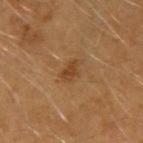No biopsy was performed on this lesion — it was imaged during a full skin examination and was not determined to be concerning. The lesion-visualizer software estimated a nevus-likeness score of about 40/100. Located on the right upper arm. Cropped from a total-body skin-imaging series; the visible field is about 15 mm. The recorded lesion diameter is about 3 mm. The tile uses cross-polarized illumination. A male patient, aged 38–42.